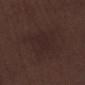{"biopsy_status": "not biopsied; imaged during a skin examination", "image": {"source": "total-body photography crop", "field_of_view_mm": 15}, "site": "left lower leg", "patient": {"sex": "male", "age_approx": 70}}